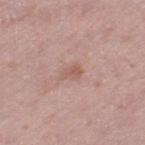  biopsy_status: not biopsied; imaged during a skin examination
  image:
    source: total-body photography crop
    field_of_view_mm: 15
  site: left thigh
  patient:
    sex: female
    age_approx: 40
  lighting: white-light
  lesion_size:
    long_diameter_mm_approx: 2.5